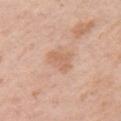Imaged during a routine full-body skin examination; the lesion was not biopsied and no histopathology is available.
A 15 mm crop from a total-body photograph taken for skin-cancer surveillance.
On the left upper arm.
The subject is a female in their mid- to late 40s.
The tile uses white-light illumination.
Longest diameter approximately 3.5 mm.